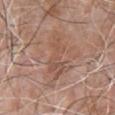Assessment:
The lesion was tiled from a total-body skin photograph and was not biopsied.
Background:
A region of skin cropped from a whole-body photographic capture, roughly 15 mm wide. From the chest. The patient is a male in their 80s.